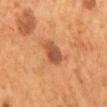<case>
<biopsy_status>not biopsied; imaged during a skin examination</biopsy_status>
<lesion_size>
  <long_diameter_mm_approx>3.5</long_diameter_mm_approx>
</lesion_size>
<automated_metrics>
  <eccentricity>0.8</eccentricity>
  <shape_asymmetry>0.15</shape_asymmetry>
  <border_irregularity_0_10>2.0</border_irregularity_0_10>
  <color_variation_0_10>5.5</color_variation_0_10>
  <peripheral_color_asymmetry>2.0</peripheral_color_asymmetry>
</automated_metrics>
<lighting>cross-polarized</lighting>
<patient>
  <sex>male</sex>
  <age_approx>55</age_approx>
</patient>
<site>mid back</site>
<image>
  <source>total-body photography crop</source>
  <field_of_view_mm>15</field_of_view_mm>
</image>
</case>